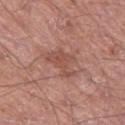<tbp_lesion>
<biopsy_status>not biopsied; imaged during a skin examination</biopsy_status>
<lesion_size>
  <long_diameter_mm_approx>4.0</long_diameter_mm_approx>
</lesion_size>
<patient>
  <sex>male</sex>
  <age_approx>70</age_approx>
</patient>
<site>leg</site>
<automated_metrics>
  <nevus_likeness_0_100>0</nevus_likeness_0_100>
  <lesion_detection_confidence_0_100>100</lesion_detection_confidence_0_100>
</automated_metrics>
<lighting>white-light</lighting>
<image>
  <source>total-body photography crop</source>
  <field_of_view_mm>15</field_of_view_mm>
</image>
</tbp_lesion>